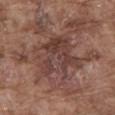Clinical impression:
Imaged during a routine full-body skin examination; the lesion was not biopsied and no histopathology is available.
Background:
Cropped from a whole-body photographic skin survey; the tile spans about 15 mm. The patient is a male in their mid- to late 70s. The lesion is located on the abdomen.